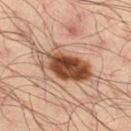Case summary:
• biopsy status · total-body-photography surveillance lesion; no biopsy
• imaging modality · total-body-photography crop, ~15 mm field of view
• site · the leg
• lesion size · ~7 mm (longest diameter)
• illumination · cross-polarized illumination
• subject · male, about 35 years old